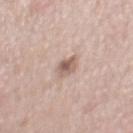Notes:
* follow-up: imaged on a skin check; not biopsied
* image: 15 mm crop, total-body photography
* patient: male, aged 48 to 52
* body site: the chest